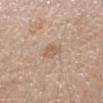<lesion>
  <biopsy_status>not biopsied; imaged during a skin examination</biopsy_status>
  <image>
    <source>total-body photography crop</source>
    <field_of_view_mm>15</field_of_view_mm>
  </image>
  <lesion_size>
    <long_diameter_mm_approx>2.5</long_diameter_mm_approx>
  </lesion_size>
  <lighting>white-light</lighting>
  <automated_metrics>
    <area_mm2_approx>3.5</area_mm2_approx>
    <shape_asymmetry>0.3</shape_asymmetry>
    <cielab_L>59</cielab_L>
    <cielab_a>18</cielab_a>
    <cielab_b>29</cielab_b>
    <vs_skin_contrast_norm>5.5</vs_skin_contrast_norm>
    <color_variation_0_10>2.0</color_variation_0_10>
    <peripheral_color_asymmetry>1.0</peripheral_color_asymmetry>
  </automated_metrics>
  <patient>
    <sex>male</sex>
    <age_approx>60</age_approx>
  </patient>
  <site>right forearm</site>
</lesion>